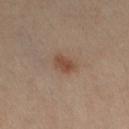- biopsy status: no biopsy performed (imaged during a skin exam)
- patient: female, aged 38 to 42
- body site: the left leg
- image: ~15 mm crop, total-body skin-cancer survey
- automated metrics: about 9 CIELAB-L* units darker than the surrounding skin and a lesion-to-skin contrast of about 7.5 (normalized; higher = more distinct); a border-irregularity index near 2/10 and internal color variation of about 3 on a 0–10 scale
- lesion diameter: ~2.5 mm (longest diameter)
- lighting: cross-polarized